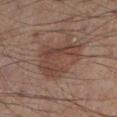| feature | finding |
|---|---|
| follow-up | catalogued during a skin exam; not biopsied |
| image-analysis metrics | a lesion area of about 20 mm², an eccentricity of roughly 0.7, and a symmetry-axis asymmetry near 0.25; a border-irregularity rating of about 3.5/10, a color-variation rating of about 4.5/10, and peripheral color asymmetry of about 1.5 |
| patient | male, in their mid- to late 50s |
| acquisition | total-body-photography crop, ~15 mm field of view |
| diameter | about 6 mm |
| illumination | cross-polarized illumination |
| body site | the leg |